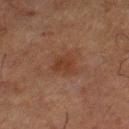follow-up = imaged on a skin check; not biopsied.